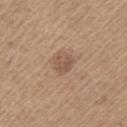biopsy status=catalogued during a skin exam; not biopsied | patient=male, aged around 65 | anatomic site=the left upper arm | image=~15 mm crop, total-body skin-cancer survey.